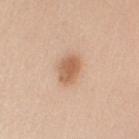Impression: The lesion was photographed on a routine skin check and not biopsied; there is no pathology result. Acquisition and patient details: The patient is a female aged 43–47. On the left upper arm. The lesion-visualizer software estimated an area of roughly 7.5 mm², an eccentricity of roughly 0.75, and two-axis asymmetry of about 0.15. It also reported border irregularity of about 1.5 on a 0–10 scale, a within-lesion color-variation index near 3.5/10, and radial color variation of about 1. A 15 mm close-up tile from a total-body photography series done for melanoma screening. The tile uses white-light illumination.